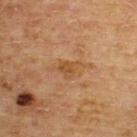Case summary:
* follow-up · imaged on a skin check; not biopsied
* location · the back
* subject · male, about 65 years old
* TBP lesion metrics · a lesion area of about 4 mm² and a shape eccentricity near 0.65
* lesion size · ~2.5 mm (longest diameter)
* acquisition · total-body-photography crop, ~15 mm field of view
* lighting · cross-polarized illumination